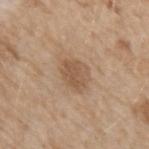| feature | finding |
|---|---|
| automated lesion analysis | a lesion-to-skin contrast of about 6 (normalized; higher = more distinct) |
| patient | male, about 45 years old |
| lighting | white-light illumination |
| acquisition | 15 mm crop, total-body photography |
| body site | the left forearm |